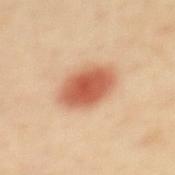{"biopsy_status": "not biopsied; imaged during a skin examination", "lighting": "cross-polarized", "site": "mid back", "lesion_size": {"long_diameter_mm_approx": 5.5}, "patient": {"sex": "female", "age_approx": 40}, "image": {"source": "total-body photography crop", "field_of_view_mm": 15}, "automated_metrics": {"cielab_L": 61, "cielab_a": 28, "cielab_b": 36, "vs_skin_darker_L": 16.0, "vs_skin_contrast_norm": 9.5, "border_irregularity_0_10": 1.5, "color_variation_0_10": 4.5, "peripheral_color_asymmetry": 1.0, "nevus_likeness_0_100": 100, "lesion_detection_confidence_0_100": 100}}